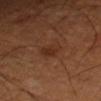Notes:
– notes · no biopsy performed (imaged during a skin exam)